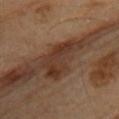notes: catalogued during a skin exam; not biopsied
image-analysis metrics: an average lesion color of about L≈33 a*≈18 b*≈26 (CIELAB), roughly 10 lightness units darker than nearby skin, and a normalized border contrast of about 9; a border-irregularity rating of about 6/10 and a peripheral color-asymmetry measure near 1; a nevus-likeness score of about 0/100 and lesion-presence confidence of about 85/100
lesion size: ~6.5 mm (longest diameter)
site: the upper back
acquisition: ~15 mm tile from a whole-body skin photo
subject: male, in their mid-80s or older